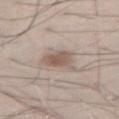Clinical impression: Part of a total-body skin-imaging series; this lesion was reviewed on a skin check and was not flagged for biopsy. Image and clinical context: The lesion's longest dimension is about 3.5 mm. A male subject, approximately 30 years of age. A 15 mm close-up tile from a total-body photography series done for melanoma screening. Captured under white-light illumination. The lesion-visualizer software estimated a shape-asymmetry score of about 0.3 (0 = symmetric). And it measured a color-variation rating of about 3/10 and a peripheral color-asymmetry measure near 1. The lesion is located on the abdomen.